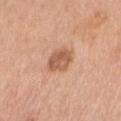Q: Was a biopsy performed?
A: total-body-photography surveillance lesion; no biopsy